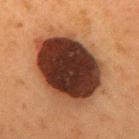Recorded during total-body skin imaging; not selected for excision or biopsy. Longest diameter approximately 9 mm. Imaged with cross-polarized lighting. The patient is a female in their 50s. From the upper back. A 15 mm close-up tile from a total-body photography series done for melanoma screening.